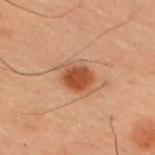| feature | finding |
|---|---|
| notes | catalogued during a skin exam; not biopsied |
| TBP lesion metrics | a lesion area of about 7 mm²; a normalized lesion–skin contrast near 9.5; internal color variation of about 2.5 on a 0–10 scale and a peripheral color-asymmetry measure near 1; a classifier nevus-likeness of about 100/100 and a detector confidence of about 100 out of 100 that the crop contains a lesion |
| size | ≈3.5 mm |
| lighting | cross-polarized illumination |
| imaging modality | ~15 mm crop, total-body skin-cancer survey |
| site | the upper back |
| subject | male, in their mid- to late 50s |